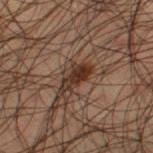{
  "biopsy_status": "not biopsied; imaged during a skin examination",
  "patient": {
    "sex": "male",
    "age_approx": 50
  },
  "site": "left thigh",
  "automated_metrics": {
    "area_mm2_approx": 5.0,
    "eccentricity": 0.9,
    "cielab_L": 22,
    "cielab_a": 14,
    "cielab_b": 20,
    "vs_skin_darker_L": 10.0,
    "vs_skin_contrast_norm": 11.0,
    "lesion_detection_confidence_0_100": 100
  },
  "image": {
    "source": "total-body photography crop",
    "field_of_view_mm": 15
  }
}